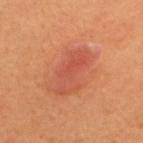Clinical impression:
The lesion was tiled from a total-body skin photograph and was not biopsied.
Context:
The lesion-visualizer software estimated a lesion area of about 13 mm², a shape eccentricity near 0.9, and two-axis asymmetry of about 0.35. It also reported a border-irregularity rating of about 4.5/10 and a within-lesion color-variation index near 5.5/10. Located on the upper back. A close-up tile cropped from a whole-body skin photograph, about 15 mm across. Captured under cross-polarized illumination. A male patient about 65 years old.